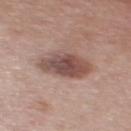notes = no biopsy performed (imaged during a skin exam)
anatomic site = the mid back
patient = male, in their mid- to late 50s
image source = total-body-photography crop, ~15 mm field of view
lesion size = about 6 mm
illumination = white-light